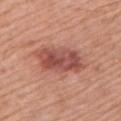- notes — imaged on a skin check; not biopsied
- lesion size — about 6.5 mm
- image — ~15 mm tile from a whole-body skin photo
- tile lighting — white-light illumination
- automated metrics — an area of roughly 17 mm², an eccentricity of roughly 0.85, and a symmetry-axis asymmetry near 0.2; a lesion color around L≈51 a*≈27 b*≈27 in CIELAB, a lesion–skin lightness drop of about 12, and a lesion-to-skin contrast of about 8.5 (normalized; higher = more distinct)
- patient — male, aged 63 to 67
- anatomic site — the left upper arm